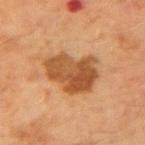Captured during whole-body skin photography for melanoma surveillance; the lesion was not biopsied. The tile uses cross-polarized illumination. The patient is a male approximately 70 years of age. Measured at roughly 5.5 mm in maximum diameter. The lesion-visualizer software estimated a footprint of about 20 mm² and an eccentricity of roughly 0.6. And it measured a border-irregularity index near 3.5/10, a within-lesion color-variation index near 4.5/10, and a peripheral color-asymmetry measure near 1.5. It also reported a nevus-likeness score of about 45/100 and a detector confidence of about 100 out of 100 that the crop contains a lesion. A 15 mm close-up tile from a total-body photography series done for melanoma screening. The lesion is on the left upper arm.